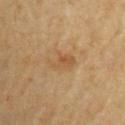follow-up: catalogued during a skin exam; not biopsied | patient: male, aged approximately 70 | lighting: cross-polarized | TBP lesion metrics: a footprint of about 5.5 mm² and an outline eccentricity of about 0.75 (0 = round, 1 = elongated); a lesion color around L≈51 a*≈17 b*≈36 in CIELAB and a lesion–skin lightness drop of about 7 | lesion diameter: about 3.5 mm | image source: ~15 mm tile from a whole-body skin photo | site: the right upper arm.